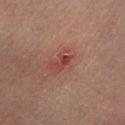Clinical impression: Captured during whole-body skin photography for melanoma surveillance; the lesion was not biopsied. Image and clinical context: The lesion-visualizer software estimated an area of roughly 3 mm², an outline eccentricity of about 0.85 (0 = round, 1 = elongated), and a shape-asymmetry score of about 0.4 (0 = symmetric). And it measured an average lesion color of about L≈37 a*≈25 b*≈23 (CIELAB), roughly 7 lightness units darker than nearby skin, and a normalized lesion–skin contrast near 7. The software also gave a border-irregularity rating of about 4.5/10 and peripheral color asymmetry of about 0. Imaged with cross-polarized lighting. A male patient, in their mid- to late 50s. A 15 mm close-up tile from a total-body photography series done for melanoma screening. From the left lower leg.